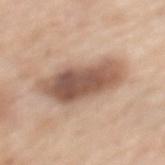Q: Was a biopsy performed?
A: imaged on a skin check; not biopsied
Q: What did automated image analysis measure?
A: an automated nevus-likeness rating near 70 out of 100
Q: Patient demographics?
A: female, aged approximately 65
Q: What lighting was used for the tile?
A: white-light
Q: What is the anatomic site?
A: the mid back
Q: How was this image acquired?
A: 15 mm crop, total-body photography
Q: How large is the lesion?
A: ≈7.5 mm